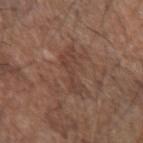Q: Is there a histopathology result?
A: catalogued during a skin exam; not biopsied
Q: What kind of image is this?
A: ~15 mm crop, total-body skin-cancer survey
Q: What are the patient's age and sex?
A: male, about 70 years old
Q: Where on the body is the lesion?
A: the left forearm